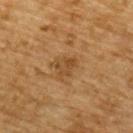Imaged during a routine full-body skin examination; the lesion was not biopsied and no histopathology is available. The patient is a male approximately 85 years of age or older. A lesion tile, about 15 mm wide, cut from a 3D total-body photograph. From the upper back. The lesion's longest dimension is about 2.5 mm. This is a cross-polarized tile.